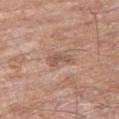Recorded during total-body skin imaging; not selected for excision or biopsy.
Cropped from a total-body skin-imaging series; the visible field is about 15 mm.
A male patient, aged around 80.
The lesion's longest dimension is about 3 mm.
On the right thigh.
This is a white-light tile.